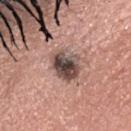follow-up — imaged on a skin check; not biopsied | location — the head or neck | image — ~15 mm tile from a whole-body skin photo | automated lesion analysis — a border-irregularity index near 2.5/10, a within-lesion color-variation index near 8.5/10, and radial color variation of about 2.5; a nevus-likeness score of about 0/100 and a lesion-detection confidence of about 100/100 | patient — female, aged around 45 | tile lighting — white-light illumination | lesion size — about 4 mm.